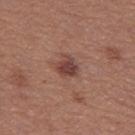• follow-up · no biopsy performed (imaged during a skin exam)
• location · the left thigh
• acquisition · ~15 mm crop, total-body skin-cancer survey
• patient · female, in their 40s